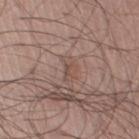Assessment: The lesion was tiled from a total-body skin photograph and was not biopsied. Image and clinical context: Imaged with white-light lighting. A 15 mm crop from a total-body photograph taken for skin-cancer surveillance. Located on the left lower leg. About 3 mm across. A male subject, about 45 years old. Automated tile analysis of the lesion measured about 7 CIELAB-L* units darker than the surrounding skin. The software also gave an automated nevus-likeness rating near 0 out of 100 and a detector confidence of about 75 out of 100 that the crop contains a lesion.